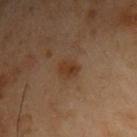Clinical impression:
Captured during whole-body skin photography for melanoma surveillance; the lesion was not biopsied.
Acquisition and patient details:
A 15 mm crop from a total-body photograph taken for skin-cancer surveillance. From the chest. The lesion's longest dimension is about 2.5 mm. This is a cross-polarized tile. The patient is a male roughly 55 years of age. The lesion-visualizer software estimated a lesion area of about 3 mm², an outline eccentricity of about 0.8 (0 = round, 1 = elongated), and a shape-asymmetry score of about 0.2 (0 = symmetric). And it measured a border-irregularity rating of about 2/10 and internal color variation of about 2 on a 0–10 scale. And it measured an automated nevus-likeness rating near 55 out of 100.